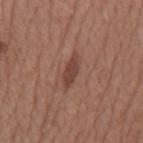Clinical impression:
This lesion was catalogued during total-body skin photography and was not selected for biopsy.
Acquisition and patient details:
This is a white-light tile. Located on the mid back. The lesion's longest dimension is about 4 mm. A male subject, aged approximately 65. A roughly 15 mm field-of-view crop from a total-body skin photograph.